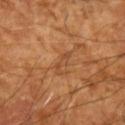Findings:
- notes — no biopsy performed (imaged during a skin exam)
- automated lesion analysis — border irregularity of about 6 on a 0–10 scale, a color-variation rating of about 0/10, and a peripheral color-asymmetry measure near 0
- imaging modality — ~15 mm crop, total-body skin-cancer survey
- subject — aged 63 to 67
- site — the left forearm